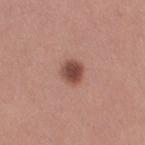Clinical impression: Part of a total-body skin-imaging series; this lesion was reviewed on a skin check and was not flagged for biopsy. Acquisition and patient details: Located on the right thigh. Longest diameter approximately 2.5 mm. The subject is a female roughly 30 years of age. Cropped from a whole-body photographic skin survey; the tile spans about 15 mm.